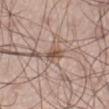Q: Was a biopsy performed?
A: total-body-photography surveillance lesion; no biopsy
Q: Who is the patient?
A: male, in their mid-40s
Q: Lesion size?
A: ~2.5 mm (longest diameter)
Q: How was this image acquired?
A: ~15 mm tile from a whole-body skin photo
Q: What is the anatomic site?
A: the left thigh
Q: What lighting was used for the tile?
A: white-light